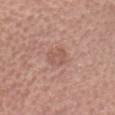{
  "lesion_size": {
    "long_diameter_mm_approx": 2.5
  },
  "site": "head or neck",
  "image": {
    "source": "total-body photography crop",
    "field_of_view_mm": 15
  },
  "patient": {
    "sex": "female",
    "age_approx": 65
  }
}